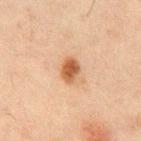  biopsy_status: not biopsied; imaged during a skin examination
  site: abdomen
  patient:
    sex: male
    age_approx: 50
  image:
    source: total-body photography crop
    field_of_view_mm: 15